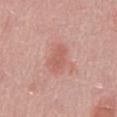Case summary:
* biopsy status: no biopsy performed (imaged during a skin exam)
* anatomic site: the front of the torso
* image-analysis metrics: an area of roughly 4 mm², an outline eccentricity of about 0.85 (0 = round, 1 = elongated), and a shape-asymmetry score of about 0.25 (0 = symmetric); a nevus-likeness score of about 20/100
* lesion diameter: about 3 mm
* tile lighting: white-light illumination
* acquisition: total-body-photography crop, ~15 mm field of view
* patient: male, approximately 50 years of age